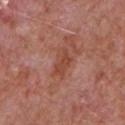{
  "biopsy_status": "not biopsied; imaged during a skin examination",
  "lesion_size": {
    "long_diameter_mm_approx": 3.5
  },
  "lighting": "white-light",
  "patient": {
    "sex": "male",
    "age_approx": 65
  },
  "automated_metrics": {
    "area_mm2_approx": 6.0,
    "eccentricity": 0.85,
    "shape_asymmetry": 0.35,
    "cielab_L": 46,
    "cielab_a": 26,
    "cielab_b": 30,
    "vs_skin_darker_L": 8.0,
    "vs_skin_contrast_norm": 6.5,
    "color_variation_0_10": 2.0,
    "peripheral_color_asymmetry": 0.5
  },
  "image": {
    "source": "total-body photography crop",
    "field_of_view_mm": 15
  },
  "site": "chest"
}